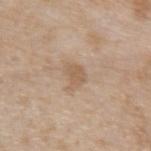  biopsy_status: not biopsied; imaged during a skin examination
  site: left forearm
  patient:
    sex: male
    age_approx: 45
  image:
    source: total-body photography crop
    field_of_view_mm: 15